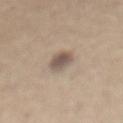{"biopsy_status": "not biopsied; imaged during a skin examination", "site": "abdomen", "image": {"source": "total-body photography crop", "field_of_view_mm": 15}, "lighting": "white-light", "automated_metrics": {"cielab_L": 54, "cielab_a": 12, "cielab_b": 23, "vs_skin_darker_L": 12.0, "nevus_likeness_0_100": 60, "lesion_detection_confidence_0_100": 100}, "patient": {"sex": "male", "age_approx": 65}, "lesion_size": {"long_diameter_mm_approx": 3.5}}